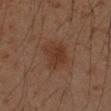This lesion was catalogued during total-body skin photography and was not selected for biopsy. Automated tile analysis of the lesion measured a border-irregularity index near 2.5/10, a color-variation rating of about 2.5/10, and a peripheral color-asymmetry measure near 1. It also reported a classifier nevus-likeness of about 20/100 and a detector confidence of about 100 out of 100 that the crop contains a lesion. A 15 mm close-up tile from a total-body photography series done for melanoma screening. From the arm. This is a cross-polarized tile. A male subject approximately 50 years of age. Approximately 3.5 mm at its widest.